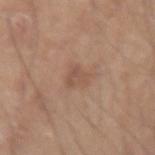Assessment: The lesion was tiled from a total-body skin photograph and was not biopsied. Acquisition and patient details: A male patient roughly 40 years of age. On the right forearm. A 15 mm crop from a total-body photograph taken for skin-cancer surveillance.